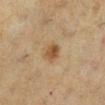The lesion was tiled from a total-body skin photograph and was not biopsied. The lesion's longest dimension is about 2.5 mm. Cropped from a total-body skin-imaging series; the visible field is about 15 mm. A female subject, aged 53 to 57. On the left lower leg. Captured under cross-polarized illumination.